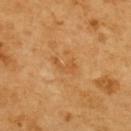Assessment:
The lesion was photographed on a routine skin check and not biopsied; there is no pathology result.
Acquisition and patient details:
Captured under cross-polarized illumination. About 3 mm across. The lesion is located on the upper back. A roughly 15 mm field-of-view crop from a total-body skin photograph. The patient is a female aged around 55.